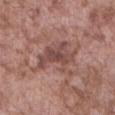The lesion was photographed on a routine skin check and not biopsied; there is no pathology result. An algorithmic analysis of the crop reported a footprint of about 16 mm², a shape eccentricity near 0.65, and two-axis asymmetry of about 0.5. The software also gave a lesion–skin lightness drop of about 10. The analysis additionally found a border-irregularity rating of about 7.5/10, a within-lesion color-variation index near 3.5/10, and a peripheral color-asymmetry measure near 1. The software also gave a nevus-likeness score of about 0/100. The subject is a male aged around 75. From the abdomen. About 5.5 mm across. A 15 mm close-up tile from a total-body photography series done for melanoma screening. Imaged with white-light lighting.